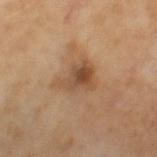Q: Was a biopsy performed?
A: catalogued during a skin exam; not biopsied
Q: How large is the lesion?
A: ≈4.5 mm
Q: What are the patient's age and sex?
A: male, in their mid-60s
Q: Illumination type?
A: cross-polarized illumination
Q: What is the imaging modality?
A: ~15 mm crop, total-body skin-cancer survey